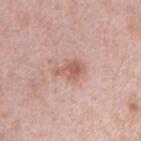Assessment: No biopsy was performed on this lesion — it was imaged during a full skin examination and was not determined to be concerning. Background: This is a white-light tile. Longest diameter approximately 3.5 mm. On the arm. A male patient aged around 40. The total-body-photography lesion software estimated a border-irregularity rating of about 4/10 and radial color variation of about 1. The software also gave a classifier nevus-likeness of about 30/100. A region of skin cropped from a whole-body photographic capture, roughly 15 mm wide.